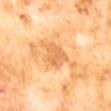{
  "biopsy_status": "not biopsied; imaged during a skin examination",
  "image": {
    "source": "total-body photography crop",
    "field_of_view_mm": 15
  },
  "patient": {
    "sex": "male",
    "age_approx": 60
  },
  "automated_metrics": {
    "area_mm2_approx": 6.0,
    "shape_asymmetry": 0.35,
    "cielab_L": 66,
    "cielab_a": 24,
    "cielab_b": 46,
    "vs_skin_darker_L": 9.0,
    "vs_skin_contrast_norm": 5.5
  },
  "site": "abdomen",
  "lesion_size": {
    "long_diameter_mm_approx": 3.0
  }
}